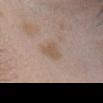Q: Was a biopsy performed?
A: imaged on a skin check; not biopsied
Q: What did automated image analysis measure?
A: an eccentricity of roughly 0.75 and a symmetry-axis asymmetry near 0.35; an average lesion color of about L≈55 a*≈15 b*≈27 (CIELAB) and about 7 CIELAB-L* units darker than the surrounding skin; border irregularity of about 4 on a 0–10 scale and a peripheral color-asymmetry measure near 0.5; an automated nevus-likeness rating near 5 out of 100
Q: How was the tile lit?
A: white-light
Q: What kind of image is this?
A: ~15 mm crop, total-body skin-cancer survey
Q: Lesion size?
A: ≈3 mm
Q: Patient demographics?
A: female, in their mid- to late 20s
Q: Where on the body is the lesion?
A: the head or neck